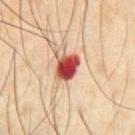Captured during whole-body skin photography for melanoma surveillance; the lesion was not biopsied. This image is a 15 mm lesion crop taken from a total-body photograph. The lesion's longest dimension is about 3.5 mm. On the front of the torso. The subject is a male approximately 50 years of age.